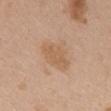Q: Was a biopsy performed?
A: catalogued during a skin exam; not biopsied
Q: What kind of image is this?
A: 15 mm crop, total-body photography
Q: What did automated image analysis measure?
A: a mean CIELAB color near L≈59 a*≈18 b*≈34, a lesion–skin lightness drop of about 7, and a normalized lesion–skin contrast near 5.5; a classifier nevus-likeness of about 5/100 and a detector confidence of about 100 out of 100 that the crop contains a lesion
Q: What is the lesion's diameter?
A: ≈5 mm
Q: How was the tile lit?
A: white-light illumination
Q: What is the anatomic site?
A: the mid back
Q: Patient demographics?
A: female, aged 63 to 67